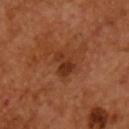Notes:
• follow-up: total-body-photography surveillance lesion; no biopsy
• acquisition: ~15 mm tile from a whole-body skin photo
• lighting: cross-polarized
• location: the chest
• subject: male, aged approximately 55
• lesion size: about 3 mm